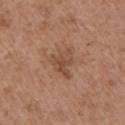No biopsy was performed on this lesion — it was imaged during a full skin examination and was not determined to be concerning. A female subject, in their mid- to late 70s. Located on the left upper arm. The total-body-photography lesion software estimated an average lesion color of about L≈48 a*≈21 b*≈30 (CIELAB) and a lesion-to-skin contrast of about 6.5 (normalized; higher = more distinct). The analysis additionally found a classifier nevus-likeness of about 0/100 and a lesion-detection confidence of about 100/100. A close-up tile cropped from a whole-body skin photograph, about 15 mm across. Approximately 4 mm at its widest. This is a white-light tile.